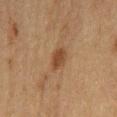workup: no biopsy performed (imaged during a skin exam)
patient: male, aged 73–77
automated metrics: an area of roughly 4.5 mm²; a border-irregularity rating of about 3/10, a within-lesion color-variation index near 2/10, and peripheral color asymmetry of about 0.5; a classifier nevus-likeness of about 100/100
acquisition: total-body-photography crop, ~15 mm field of view
site: the front of the torso
lesion diameter: ~3.5 mm (longest diameter)